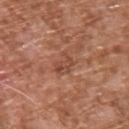biopsy status = total-body-photography surveillance lesion; no biopsy
size = about 3 mm
imaging modality = total-body-photography crop, ~15 mm field of view
subject = male, roughly 75 years of age
body site = the upper back